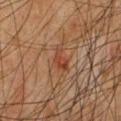Findings:
– follow-up · no biopsy performed (imaged during a skin exam)
– body site · the chest
– image source · ~15 mm crop, total-body skin-cancer survey
– lesion diameter · about 3.5 mm
– image-analysis metrics · a lesion color around L≈35 a*≈20 b*≈27 in CIELAB and about 6 CIELAB-L* units darker than the surrounding skin; internal color variation of about 2.5 on a 0–10 scale
– patient · male, approximately 50 years of age
– illumination · cross-polarized